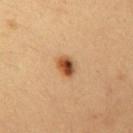workup: catalogued during a skin exam; not biopsied | subject: female, in their mid-30s | lesion size: ~2.5 mm (longest diameter) | automated metrics: a lesion area of about 5 mm², a shape eccentricity near 0.55, and a shape-asymmetry score of about 0.2 (0 = symmetric); border irregularity of about 1.5 on a 0–10 scale and a within-lesion color-variation index near 9.5/10 | image: ~15 mm tile from a whole-body skin photo | body site: the left upper arm.